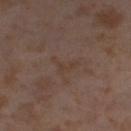Impression:
Imaged during a routine full-body skin examination; the lesion was not biopsied and no histopathology is available.
Background:
Automated tile analysis of the lesion measured an outline eccentricity of about 0.75 (0 = round, 1 = elongated) and two-axis asymmetry of about 0.7. It also reported border irregularity of about 7.5 on a 0–10 scale and a peripheral color-asymmetry measure near 0. A 15 mm crop from a total-body photograph taken for skin-cancer surveillance. Captured under cross-polarized illumination. Longest diameter approximately 3 mm. A female subject, approximately 55 years of age. On the right thigh.